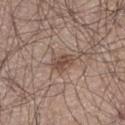– workup — imaged on a skin check; not biopsied
– subject — male, approximately 65 years of age
– site — the leg
– automated metrics — a lesion area of about 5 mm², a shape eccentricity near 0.7, and two-axis asymmetry of about 0.25; an average lesion color of about L≈48 a*≈17 b*≈25 (CIELAB), a lesion–skin lightness drop of about 10, and a normalized border contrast of about 7.5
– lighting — white-light illumination
– acquisition — 15 mm crop, total-body photography
– lesion size — ~3 mm (longest diameter)